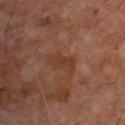Notes:
* biopsy status: total-body-photography surveillance lesion; no biopsy
* size: ≈3.5 mm
* TBP lesion metrics: a lesion area of about 5.5 mm², an outline eccentricity of about 0.85 (0 = round, 1 = elongated), and two-axis asymmetry of about 0.3; an automated nevus-likeness rating near 0 out of 100 and a detector confidence of about 100 out of 100 that the crop contains a lesion
* lighting: cross-polarized illumination
* image source: total-body-photography crop, ~15 mm field of view
* subject: male, roughly 65 years of age